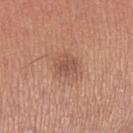Recorded during total-body skin imaging; not selected for excision or biopsy. Imaged with white-light lighting. About 3 mm across. A female subject, aged 23 to 27. On the right upper arm. A region of skin cropped from a whole-body photographic capture, roughly 15 mm wide.